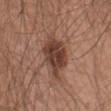This lesion was catalogued during total-body skin photography and was not selected for biopsy. Approximately 6 mm at its widest. Automated image analysis of the tile measured an area of roughly 15 mm² and a shape-asymmetry score of about 0.25 (0 = symmetric). And it measured a border-irregularity index near 3/10, a within-lesion color-variation index near 4/10, and a peripheral color-asymmetry measure near 1. Captured under white-light illumination. The patient is a male aged approximately 65. A 15 mm crop from a total-body photograph taken for skin-cancer surveillance. The lesion is on the abdomen.